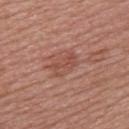imaging modality: ~15 mm crop, total-body skin-cancer survey; lighting: white-light illumination; location: the upper back; patient: female, in their mid- to late 50s.